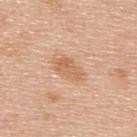The subject is a male in their 50s. The tile uses white-light illumination. On the upper back. A 15 mm close-up extracted from a 3D total-body photography capture. The lesion-visualizer software estimated an eccentricity of roughly 0.85 and a shape-asymmetry score of about 0.25 (0 = symmetric). It also reported an average lesion color of about L≈63 a*≈21 b*≈36 (CIELAB) and roughly 9 lightness units darker than nearby skin. And it measured a border-irregularity index near 3/10 and a peripheral color-asymmetry measure near 1. The recorded lesion diameter is about 4 mm.